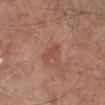No biopsy was performed on this lesion — it was imaged during a full skin examination and was not determined to be concerning. A male patient, approximately 50 years of age. The lesion is on the left leg. The lesion-visualizer software estimated an area of roughly 3 mm², an eccentricity of roughly 0.85, and a shape-asymmetry score of about 0.25 (0 = symmetric). The software also gave a normalized border contrast of about 5.5. The analysis additionally found border irregularity of about 2.5 on a 0–10 scale, a color-variation rating of about 0.5/10, and a peripheral color-asymmetry measure near 0. The software also gave an automated nevus-likeness rating near 10 out of 100. Cropped from a total-body skin-imaging series; the visible field is about 15 mm.